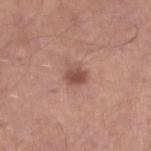biopsy status — imaged on a skin check; not biopsied
patient — male, roughly 50 years of age
body site — the right lower leg
diameter — about 3 mm
tile lighting — white-light illumination
imaging modality — total-body-photography crop, ~15 mm field of view